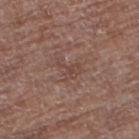{
  "biopsy_status": "not biopsied; imaged during a skin examination",
  "patient": {
    "sex": "female",
    "age_approx": 80
  },
  "lesion_size": {
    "long_diameter_mm_approx": 3.0
  },
  "automated_metrics": {
    "border_irregularity_0_10": 8.0,
    "peripheral_color_asymmetry": 0.5,
    "lesion_detection_confidence_0_100": 95
  },
  "image": {
    "source": "total-body photography crop",
    "field_of_view_mm": 15
  },
  "site": "left thigh",
  "lighting": "white-light"
}